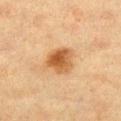Q: Was this lesion biopsied?
A: catalogued during a skin exam; not biopsied
Q: What is the anatomic site?
A: the leg
Q: How was this image acquired?
A: ~15 mm tile from a whole-body skin photo
Q: Patient demographics?
A: female, aged 38–42